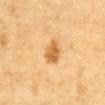Part of a total-body skin-imaging series; this lesion was reviewed on a skin check and was not flagged for biopsy.
Cropped from a total-body skin-imaging series; the visible field is about 15 mm.
The patient is a male in their mid-80s or older.
On the abdomen.